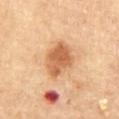workup: catalogued during a skin exam; not biopsied | automated lesion analysis: a lesion area of about 12 mm², an eccentricity of roughly 0.7, and a shape-asymmetry score of about 0.15 (0 = symmetric); a mean CIELAB color near L≈60 a*≈24 b*≈38 and roughly 13 lightness units darker than nearby skin | lesion size: ~5 mm (longest diameter) | site: the front of the torso | subject: male, aged approximately 85 or older | illumination: cross-polarized illumination | image source: 15 mm crop, total-body photography.